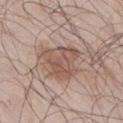- follow-up · catalogued during a skin exam; not biopsied
- size · about 5 mm
- imaging modality · ~15 mm crop, total-body skin-cancer survey
- anatomic site · the right thigh
- TBP lesion metrics · a symmetry-axis asymmetry near 0.35; a border-irregularity rating of about 4/10, a color-variation rating of about 4/10, and a peripheral color-asymmetry measure near 1.5; a detector confidence of about 100 out of 100 that the crop contains a lesion
- patient · male, in their 70s
- illumination · white-light illumination